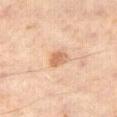{
  "biopsy_status": "not biopsied; imaged during a skin examination",
  "site": "right thigh",
  "lighting": "cross-polarized",
  "patient": {
    "sex": "male",
    "age_approx": 60
  },
  "image": {
    "source": "total-body photography crop",
    "field_of_view_mm": 15
  }
}